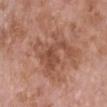Imaged during a routine full-body skin examination; the lesion was not biopsied and no histopathology is available.
The lesion-visualizer software estimated a normalized border contrast of about 6.5. It also reported border irregularity of about 8.5 on a 0–10 scale and a color-variation rating of about 4/10. The analysis additionally found a nevus-likeness score of about 0/100.
The lesion is located on the chest.
A region of skin cropped from a whole-body photographic capture, roughly 15 mm wide.
A female patient aged 73 to 77.
Imaged with white-light lighting.